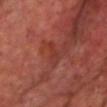biopsy status: catalogued during a skin exam; not biopsied
subject: male, aged around 70
size: about 3 mm
location: the chest
image: total-body-photography crop, ~15 mm field of view
illumination: cross-polarized illumination